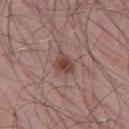Impression:
This lesion was catalogued during total-body skin photography and was not selected for biopsy.
Background:
Longest diameter approximately 3 mm. Automated image analysis of the tile measured an average lesion color of about L≈44 a*≈20 b*≈23 (CIELAB) and a normalized lesion–skin contrast near 8. And it measured a nevus-likeness score of about 85/100 and a detector confidence of about 100 out of 100 that the crop contains a lesion. The lesion is located on the leg. A male subject aged 53 to 57. A region of skin cropped from a whole-body photographic capture, roughly 15 mm wide. The tile uses white-light illumination.